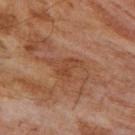{"biopsy_status": "not biopsied; imaged during a skin examination", "patient": {"sex": "male", "age_approx": 55}, "image": {"source": "total-body photography crop", "field_of_view_mm": 15}, "lesion_size": {"long_diameter_mm_approx": 3.0}, "automated_metrics": {"cielab_L": 44, "cielab_a": 24, "cielab_b": 34, "vs_skin_darker_L": 6.0, "vs_skin_contrast_norm": 5.5, "border_irregularity_0_10": 6.0, "color_variation_0_10": 0.0, "peripheral_color_asymmetry": 0.0}, "lighting": "cross-polarized", "site": "right upper arm"}